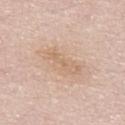The lesion was photographed on a routine skin check and not biopsied; there is no pathology result. Cropped from a total-body skin-imaging series; the visible field is about 15 mm. A male subject, in their mid- to late 60s. The lesion is on the upper back. The lesion's longest dimension is about 5.5 mm.